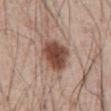Q: Is there a histopathology result?
A: catalogued during a skin exam; not biopsied
Q: Patient demographics?
A: male, about 55 years old
Q: Where on the body is the lesion?
A: the abdomen
Q: Illumination type?
A: white-light
Q: Automated lesion metrics?
A: a border-irregularity rating of about 2/10 and radial color variation of about 1.5
Q: Lesion size?
A: ~4 mm (longest diameter)
Q: What kind of image is this?
A: ~15 mm tile from a whole-body skin photo